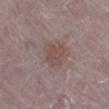Findings:
• biopsy status: catalogued during a skin exam; not biopsied
• image source: ~15 mm tile from a whole-body skin photo
• automated lesion analysis: a border-irregularity rating of about 2.5/10, a color-variation rating of about 2/10, and peripheral color asymmetry of about 0.5; an automated nevus-likeness rating near 30 out of 100
• location: the left lower leg
• subject: female, approximately 65 years of age
• lighting: white-light